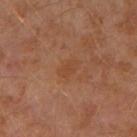A male subject in their 30s.
A 15 mm close-up tile from a total-body photography series done for melanoma screening.
Approximately 2.5 mm at its widest.
On the arm.